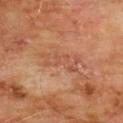Clinical impression:
No biopsy was performed on this lesion — it was imaged during a full skin examination and was not determined to be concerning.
Clinical summary:
Located on the chest. Approximately 5 mm at its widest. This is a cross-polarized tile. The total-body-photography lesion software estimated a mean CIELAB color near L≈50 a*≈24 b*≈32, about 6 CIELAB-L* units darker than the surrounding skin, and a normalized lesion–skin contrast near 4.5. It also reported a border-irregularity rating of about 8/10, internal color variation of about 1.5 on a 0–10 scale, and peripheral color asymmetry of about 0.5. The software also gave a nevus-likeness score of about 0/100. A region of skin cropped from a whole-body photographic capture, roughly 15 mm wide. A male subject aged 58–62.